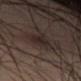Captured during whole-body skin photography for melanoma surveillance; the lesion was not biopsied. The patient is a male about 40 years old. The tile uses cross-polarized illumination. Cropped from a whole-body photographic skin survey; the tile spans about 15 mm. On the right thigh. Approximately 3.5 mm at its widest.